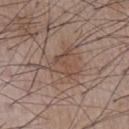Clinical impression:
Captured during whole-body skin photography for melanoma surveillance; the lesion was not biopsied.
Background:
The lesion is on the chest. A male patient, roughly 55 years of age. Cropped from a whole-body photographic skin survey; the tile spans about 15 mm. The total-body-photography lesion software estimated an outline eccentricity of about 0.45 (0 = round, 1 = elongated) and a symmetry-axis asymmetry near 0.55. It also reported a lesion color around L≈48 a*≈17 b*≈25 in CIELAB, about 6 CIELAB-L* units darker than the surrounding skin, and a normalized border contrast of about 5. And it measured a classifier nevus-likeness of about 5/100 and a lesion-detection confidence of about 90/100. Measured at roughly 3.5 mm in maximum diameter. Imaged with white-light lighting.